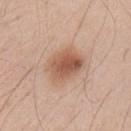Captured during whole-body skin photography for melanoma surveillance; the lesion was not biopsied. The tile uses white-light illumination. Located on the left upper arm. A male patient, in their mid-40s. A roughly 15 mm field-of-view crop from a total-body skin photograph. Longest diameter approximately 4.5 mm. Automated tile analysis of the lesion measured a footprint of about 13 mm², an outline eccentricity of about 0.65 (0 = round, 1 = elongated), and a symmetry-axis asymmetry near 0.15.